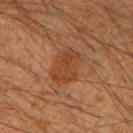{
  "biopsy_status": "not biopsied; imaged during a skin examination",
  "lesion_size": {
    "long_diameter_mm_approx": 4.5
  },
  "image": {
    "source": "total-body photography crop",
    "field_of_view_mm": 15
  },
  "site": "right upper arm",
  "patient": {
    "sex": "male",
    "age_approx": 35
  },
  "lighting": "cross-polarized"
}